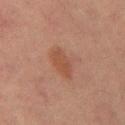  biopsy_status: not biopsied; imaged during a skin examination
  site: front of the torso
  lighting: cross-polarized
  patient:
    sex: male
    age_approx: 65
  image:
    source: total-body photography crop
    field_of_view_mm: 15
  lesion_size:
    long_diameter_mm_approx: 3.5